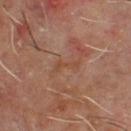No biopsy was performed on this lesion — it was imaged during a full skin examination and was not determined to be concerning. A male patient, aged approximately 60. On the chest. About 3 mm across. This is a cross-polarized tile. An algorithmic analysis of the crop reported a mean CIELAB color near L≈42 a*≈20 b*≈28 and about 5 CIELAB-L* units darker than the surrounding skin. The software also gave a border-irregularity rating of about 6.5/10, internal color variation of about 0 on a 0–10 scale, and peripheral color asymmetry of about 0. It also reported a nevus-likeness score of about 0/100 and a lesion-detection confidence of about 100/100. A close-up tile cropped from a whole-body skin photograph, about 15 mm across.